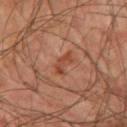Notes:
– biopsy status: imaged on a skin check; not biopsied
– acquisition: ~15 mm crop, total-body skin-cancer survey
– patient: male, aged around 75
– automated lesion analysis: a lesion area of about 3.5 mm², an eccentricity of roughly 0.9, and two-axis asymmetry of about 0.5; radial color variation of about 0; a classifier nevus-likeness of about 0/100 and a detector confidence of about 100 out of 100 that the crop contains a lesion
– diameter: about 3.5 mm
– anatomic site: the left upper arm